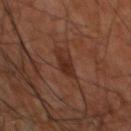notes = total-body-photography surveillance lesion; no biopsy | acquisition = ~15 mm tile from a whole-body skin photo | subject = male, about 60 years old | anatomic site = the upper back.